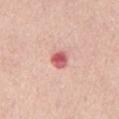biopsy status=imaged on a skin check; not biopsied
anatomic site=the abdomen
patient=male, roughly 50 years of age
lesion size=≈2.5 mm
illumination=white-light illumination
imaging modality=total-body-photography crop, ~15 mm field of view